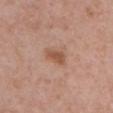Clinical impression:
Captured during whole-body skin photography for melanoma surveillance; the lesion was not biopsied.
Image and clinical context:
The subject is a female in their 60s. Cropped from a total-body skin-imaging series; the visible field is about 15 mm. On the chest. An algorithmic analysis of the crop reported a footprint of about 4 mm² and a shape eccentricity near 0.85.